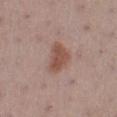{"biopsy_status": "not biopsied; imaged during a skin examination", "image": {"source": "total-body photography crop", "field_of_view_mm": 15}, "lesion_size": {"long_diameter_mm_approx": 4.0}, "lighting": "white-light", "patient": {"sex": "female", "age_approx": 55}, "site": "left lower leg"}